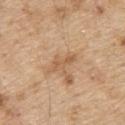biopsy status: catalogued during a skin exam; not biopsied | image source: total-body-photography crop, ~15 mm field of view | illumination: white-light | lesion diameter: ≈3 mm | body site: the upper back | patient: male, aged around 70.